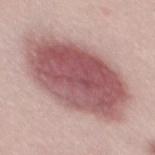<tbp_lesion>
<biopsy_status>not biopsied; imaged during a skin examination</biopsy_status>
<automated_metrics>
  <nevus_likeness_0_100>90</nevus_likeness_0_100>
  <lesion_detection_confidence_0_100>100</lesion_detection_confidence_0_100>
</automated_metrics>
<image>
  <source>total-body photography crop</source>
  <field_of_view_mm>15</field_of_view_mm>
</image>
<lesion_size>
  <long_diameter_mm_approx>11.0</long_diameter_mm_approx>
</lesion_size>
<lighting>white-light</lighting>
<patient>
  <sex>male</sex>
  <age_approx>30</age_approx>
</patient>
</tbp_lesion>